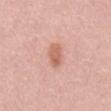<tbp_lesion>
  <biopsy_status>not biopsied; imaged during a skin examination</biopsy_status>
  <lighting>white-light</lighting>
  <patient>
    <sex>male</sex>
    <age_approx>55</age_approx>
  </patient>
  <lesion_size>
    <long_diameter_mm_approx>3.0</long_diameter_mm_approx>
  </lesion_size>
  <image>
    <source>total-body photography crop</source>
    <field_of_view_mm>15</field_of_view_mm>
  </image>
  <site>mid back</site>
</tbp_lesion>